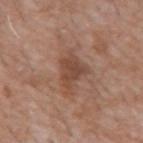Image and clinical context: A male patient, aged around 60. The lesion-visualizer software estimated a mean CIELAB color near L≈46 a*≈20 b*≈28, about 9 CIELAB-L* units darker than the surrounding skin, and a normalized border contrast of about 7. A 15 mm close-up extracted from a 3D total-body photography capture. On the chest. Captured under white-light illumination. The recorded lesion diameter is about 5 mm.